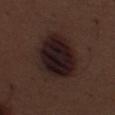{"biopsy_status": "not biopsied; imaged during a skin examination", "image": {"source": "total-body photography crop", "field_of_view_mm": 15}, "site": "leg", "patient": {"sex": "male", "age_approx": 70}}